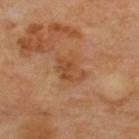notes: imaged on a skin check; not biopsied
anatomic site: the upper back
image: ~15 mm crop, total-body skin-cancer survey
subject: male, about 70 years old
tile lighting: cross-polarized
automated lesion analysis: an average lesion color of about L≈46 a*≈22 b*≈35 (CIELAB), about 7 CIELAB-L* units darker than the surrounding skin, and a lesion-to-skin contrast of about 6 (normalized; higher = more distinct); border irregularity of about 3.5 on a 0–10 scale, internal color variation of about 3.5 on a 0–10 scale, and a peripheral color-asymmetry measure near 1; a nevus-likeness score of about 0/100